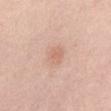Impression: Part of a total-body skin-imaging series; this lesion was reviewed on a skin check and was not flagged for biopsy. Image and clinical context: A region of skin cropped from a whole-body photographic capture, roughly 15 mm wide. A male subject, aged approximately 60. The lesion is on the abdomen.